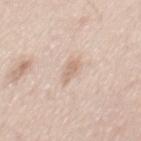site = the mid back | image = 15 mm crop, total-body photography | lesion diameter = ~3 mm (longest diameter) | illumination = white-light illumination | subject = male, aged 43 to 47 | image-analysis metrics = a lesion area of about 3 mm² and an eccentricity of roughly 0.9; a nevus-likeness score of about 0/100 and a detector confidence of about 95 out of 100 that the crop contains a lesion.